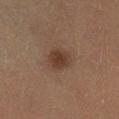Captured during whole-body skin photography for melanoma surveillance; the lesion was not biopsied.
The subject is a male in their mid-50s.
An algorithmic analysis of the crop reported a footprint of about 6 mm², an outline eccentricity of about 0.5 (0 = round, 1 = elongated), and a symmetry-axis asymmetry near 0.15. The analysis additionally found a border-irregularity rating of about 1.5/10, a color-variation rating of about 2/10, and peripheral color asymmetry of about 0.5. The analysis additionally found a detector confidence of about 100 out of 100 that the crop contains a lesion.
Located on the right lower leg.
Captured under cross-polarized illumination.
About 3 mm across.
A lesion tile, about 15 mm wide, cut from a 3D total-body photograph.